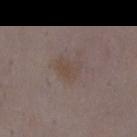biopsy_status: not biopsied; imaged during a skin examination
patient:
  sex: female
  age_approx: 35
site: abdomen
lighting: white-light
image:
  source: total-body photography crop
  field_of_view_mm: 15
lesion_size:
  long_diameter_mm_approx: 3.0
automated_metrics:
  border_irregularity_0_10: 3.0
  color_variation_0_10: 2.5
  peripheral_color_asymmetry: 1.0